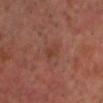<record>
  <biopsy_status>not biopsied; imaged during a skin examination</biopsy_status>
  <image>
    <source>total-body photography crop</source>
    <field_of_view_mm>15</field_of_view_mm>
  </image>
  <site>head or neck</site>
  <lesion_size>
    <long_diameter_mm_approx>2.5</long_diameter_mm_approx>
  </lesion_size>
  <patient>
    <sex>male</sex>
    <age_approx>55</age_approx>
  </patient>
  <lighting>cross-polarized</lighting>
</record>